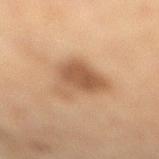Case summary:
– workup · no biopsy performed (imaged during a skin exam)
– imaging modality · 15 mm crop, total-body photography
– size · about 5.5 mm
– anatomic site · the right lower leg
– patient · female, in their mid-50s